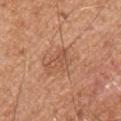Captured during whole-body skin photography for melanoma surveillance; the lesion was not biopsied.
From the right upper arm.
Imaged with white-light lighting.
A male patient roughly 30 years of age.
A lesion tile, about 15 mm wide, cut from a 3D total-body photograph.
The recorded lesion diameter is about 3.5 mm.